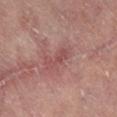Assessment: Part of a total-body skin-imaging series; this lesion was reviewed on a skin check and was not flagged for biopsy. Acquisition and patient details: A lesion tile, about 15 mm wide, cut from a 3D total-body photograph. A female subject, about 75 years old. The lesion is located on the left lower leg. Longest diameter approximately 3.5 mm. An algorithmic analysis of the crop reported an area of roughly 4 mm², a shape eccentricity near 0.95, and two-axis asymmetry of about 0.4. And it measured a border-irregularity index near 5/10 and a color-variation rating of about 1/10. The software also gave a detector confidence of about 100 out of 100 that the crop contains a lesion. Imaged with cross-polarized lighting.